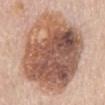Context: Located on the chest. Imaged with white-light lighting. A 15 mm close-up extracted from a 3D total-body photography capture. Automated tile analysis of the lesion measured a lesion–skin lightness drop of about 17. The analysis additionally found border irregularity of about 1.5 on a 0–10 scale, a within-lesion color-variation index near 10/10, and peripheral color asymmetry of about 3.5. A female patient aged around 65.